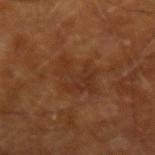Clinical impression:
This lesion was catalogued during total-body skin photography and was not selected for biopsy.
Acquisition and patient details:
Automated tile analysis of the lesion measured an outline eccentricity of about 0.7 (0 = round, 1 = elongated). The analysis additionally found roughly 5 lightness units darker than nearby skin and a lesion-to-skin contrast of about 5.5 (normalized; higher = more distinct). The analysis additionally found a border-irregularity index near 6.5/10, a color-variation rating of about 3.5/10, and a peripheral color-asymmetry measure near 1. Longest diameter approximately 4.5 mm. Captured under cross-polarized illumination. Cropped from a total-body skin-imaging series; the visible field is about 15 mm. From the arm. A male patient, in their mid- to late 60s.